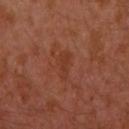{
  "biopsy_status": "not biopsied; imaged during a skin examination",
  "image": {
    "source": "total-body photography crop",
    "field_of_view_mm": 15
  },
  "lighting": "cross-polarized",
  "site": "left upper arm",
  "patient": {
    "sex": "male",
    "age_approx": 30
  },
  "lesion_size": {
    "long_diameter_mm_approx": 3.0
  },
  "automated_metrics": {
    "area_mm2_approx": 4.5,
    "eccentricity": 0.85,
    "cielab_L": 35,
    "cielab_a": 25,
    "cielab_b": 31,
    "vs_skin_contrast_norm": 5.5,
    "nevus_likeness_0_100": 0,
    "lesion_detection_confidence_0_100": 100
  }
}